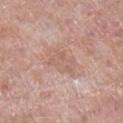The lesion was photographed on a routine skin check and not biopsied; there is no pathology result. A male subject aged 73 to 77. The recorded lesion diameter is about 4 mm. The total-body-photography lesion software estimated a lesion–skin lightness drop of about 7 and a normalized border contrast of about 4.5. And it measured a border-irregularity index near 3/10 and a peripheral color-asymmetry measure near 1. The analysis additionally found a classifier nevus-likeness of about 0/100 and lesion-presence confidence of about 100/100. Located on the right lower leg. Imaged with white-light lighting. A roughly 15 mm field-of-view crop from a total-body skin photograph.